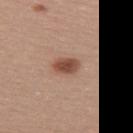{"biopsy_status": "not biopsied; imaged during a skin examination", "image": {"source": "total-body photography crop", "field_of_view_mm": 15}, "patient": {"sex": "female", "age_approx": 25}, "site": "upper back"}